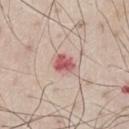<case>
<biopsy_status>not biopsied; imaged during a skin examination</biopsy_status>
<lesion_size>
  <long_diameter_mm_approx>2.5</long_diameter_mm_approx>
</lesion_size>
<lighting>white-light</lighting>
<site>front of the torso</site>
<image>
  <source>total-body photography crop</source>
  <field_of_view_mm>15</field_of_view_mm>
</image>
<patient>
  <sex>male</sex>
  <age_approx>80</age_approx>
</patient>
</case>